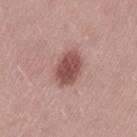No biopsy was performed on this lesion — it was imaged during a full skin examination and was not determined to be concerning. A 15 mm crop from a total-body photograph taken for skin-cancer surveillance. The subject is a female aged 48 to 52. From the left thigh.